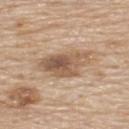– follow-up · no biopsy performed (imaged during a skin exam)
– body site · the upper back
– automated metrics · an area of roughly 14 mm², a shape eccentricity near 0.8, and a shape-asymmetry score of about 0.3 (0 = symmetric); a mean CIELAB color near L≈56 a*≈17 b*≈31, roughly 12 lightness units darker than nearby skin, and a lesion-to-skin contrast of about 8 (normalized; higher = more distinct); a border-irregularity index near 3.5/10, a within-lesion color-variation index near 7.5/10, and peripheral color asymmetry of about 3; a lesion-detection confidence of about 100/100
– patient · male, approximately 80 years of age
– imaging modality · ~15 mm crop, total-body skin-cancer survey